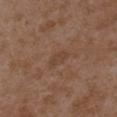  biopsy_status: not biopsied; imaged during a skin examination
  image:
    source: total-body photography crop
    field_of_view_mm: 15
  lighting: white-light
  lesion_size:
    long_diameter_mm_approx: 3.0
  site: arm
  patient:
    sex: female
    age_approx: 35